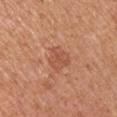The lesion was tiled from a total-body skin photograph and was not biopsied.
A male patient aged approximately 55.
This is a white-light tile.
Automated image analysis of the tile measured an eccentricity of roughly 0.55 and a symmetry-axis asymmetry near 0.25. The software also gave a lesion color around L≈53 a*≈26 b*≈33 in CIELAB. The software also gave border irregularity of about 2.5 on a 0–10 scale, internal color variation of about 2 on a 0–10 scale, and radial color variation of about 0.5. The software also gave a lesion-detection confidence of about 100/100.
From the left upper arm.
This image is a 15 mm lesion crop taken from a total-body photograph.
Longest diameter approximately 3 mm.